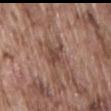{"biopsy_status": "not biopsied; imaged during a skin examination", "site": "lower back", "lesion_size": {"long_diameter_mm_approx": 3.0}, "patient": {"sex": "male", "age_approx": 75}, "image": {"source": "total-body photography crop", "field_of_view_mm": 15}}